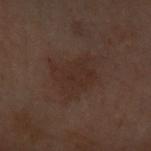Case summary:
• notes — total-body-photography surveillance lesion; no biopsy
• patient — female, approximately 60 years of age
• diameter — ≈5.5 mm
• illumination — cross-polarized illumination
• TBP lesion metrics — a classifier nevus-likeness of about 15/100 and a lesion-detection confidence of about 100/100
• acquisition — total-body-photography crop, ~15 mm field of view
• anatomic site — the front of the torso